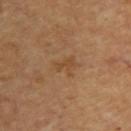Q: Was this lesion biopsied?
A: no biopsy performed (imaged during a skin exam)
Q: Automated lesion metrics?
A: a footprint of about 5.5 mm², an eccentricity of roughly 0.65, and a shape-asymmetry score of about 0.4 (0 = symmetric); a mean CIELAB color near L≈47 a*≈19 b*≈35 and a normalized border contrast of about 5; a border-irregularity rating of about 4.5/10; a classifier nevus-likeness of about 0/100 and lesion-presence confidence of about 100/100
Q: How was the tile lit?
A: cross-polarized
Q: How large is the lesion?
A: ~3 mm (longest diameter)
Q: Who is the patient?
A: in their 60s
Q: What is the anatomic site?
A: the upper back
Q: How was this image acquired?
A: total-body-photography crop, ~15 mm field of view